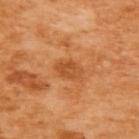Case summary:
• follow-up: catalogued during a skin exam; not biopsied
• site: the upper back
• imaging modality: 15 mm crop, total-body photography
• lesion size: ~3 mm (longest diameter)
• patient: female, aged around 55